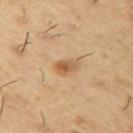– workup: imaged on a skin check; not biopsied
– automated metrics: a footprint of about 4 mm², a shape eccentricity near 0.75, and a shape-asymmetry score of about 0.2 (0 = symmetric); a nevus-likeness score of about 85/100 and a detector confidence of about 100 out of 100 that the crop contains a lesion
– site: the upper back
– subject: male, in their mid- to late 50s
– acquisition: ~15 mm crop, total-body skin-cancer survey
– lighting: cross-polarized
– size: about 2.5 mm